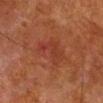The lesion was photographed on a routine skin check and not biopsied; there is no pathology result.